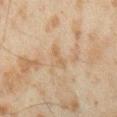| field | value |
|---|---|
| workup | imaged on a skin check; not biopsied |
| diameter | ≈2.5 mm |
| location | the right forearm |
| subject | male, about 45 years old |
| automated lesion analysis | an area of roughly 2.5 mm², an outline eccentricity of about 0.9 (0 = round, 1 = elongated), and a shape-asymmetry score of about 0.4 (0 = symmetric); border irregularity of about 4.5 on a 0–10 scale, a within-lesion color-variation index near 0/10, and peripheral color asymmetry of about 0; a nevus-likeness score of about 0/100 and a detector confidence of about 100 out of 100 that the crop contains a lesion |
| image | total-body-photography crop, ~15 mm field of view |
| illumination | cross-polarized |